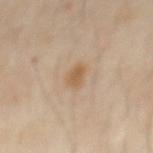No biopsy was performed on this lesion — it was imaged during a full skin examination and was not determined to be concerning. This is a cross-polarized tile. Located on the abdomen. The subject is a male in their 60s. An algorithmic analysis of the crop reported an area of roughly 4 mm², an outline eccentricity of about 0.7 (0 = round, 1 = elongated), and a shape-asymmetry score of about 0.2 (0 = symmetric). A 15 mm crop from a total-body photograph taken for skin-cancer surveillance.